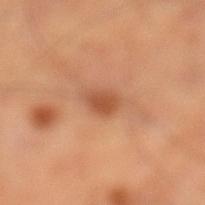Case summary:
• notes: imaged on a skin check; not biopsied
• lesion size: about 2.5 mm
• body site: the left lower leg
• subject: male, aged 58 to 62
• image source: ~15 mm tile from a whole-body skin photo
• illumination: cross-polarized